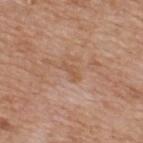Assessment: Captured during whole-body skin photography for melanoma surveillance; the lesion was not biopsied. Acquisition and patient details: The lesion is located on the upper back. Longest diameter approximately 2.5 mm. The tile uses white-light illumination. A 15 mm close-up extracted from a 3D total-body photography capture. A male patient roughly 60 years of age.